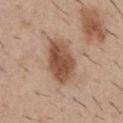| field | value |
|---|---|
| follow-up | no biopsy performed (imaged during a skin exam) |
| tile lighting | white-light illumination |
| subject | male, in their 30s |
| lesion diameter | ≈5.5 mm |
| site | the abdomen |
| image source | 15 mm crop, total-body photography |